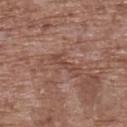workup: catalogued during a skin exam; not biopsied | location: the upper back | acquisition: ~15 mm crop, total-body skin-cancer survey | illumination: white-light illumination | subject: female, aged around 75.